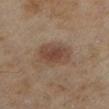Captured during whole-body skin photography for melanoma surveillance; the lesion was not biopsied. Captured under cross-polarized illumination. On the left lower leg. A female patient aged around 60. A lesion tile, about 15 mm wide, cut from a 3D total-body photograph. The lesion-visualizer software estimated a lesion area of about 12 mm², a shape eccentricity near 0.65, and a shape-asymmetry score of about 0.15 (0 = symmetric). And it measured a lesion–skin lightness drop of about 8 and a normalized lesion–skin contrast near 7. And it measured border irregularity of about 2 on a 0–10 scale, a within-lesion color-variation index near 4/10, and a peripheral color-asymmetry measure near 1. About 4.5 mm across.